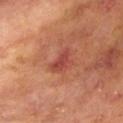follow-up: catalogued during a skin exam; not biopsied | size: ~3.5 mm (longest diameter) | lighting: cross-polarized | anatomic site: the right upper arm | imaging modality: ~15 mm crop, total-body skin-cancer survey | patient: female, about 75 years old.